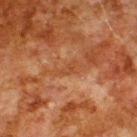The lesion was photographed on a routine skin check and not biopsied; there is no pathology result. Longest diameter approximately 2.5 mm. A male subject, about 80 years old. Captured under cross-polarized illumination. On the upper back. A close-up tile cropped from a whole-body skin photograph, about 15 mm across. The lesion-visualizer software estimated a lesion area of about 2 mm², an eccentricity of roughly 0.9, and two-axis asymmetry of about 0.45. And it measured internal color variation of about 0 on a 0–10 scale. The software also gave an automated nevus-likeness rating near 0 out of 100 and lesion-presence confidence of about 55/100.